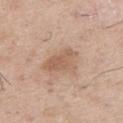* notes · catalogued during a skin exam; not biopsied
* illumination · white-light
* patient · male, roughly 50 years of age
* diameter · ~5 mm (longest diameter)
* automated metrics · a border-irregularity rating of about 3.5/10, internal color variation of about 3 on a 0–10 scale, and a peripheral color-asymmetry measure near 1; an automated nevus-likeness rating near 5 out of 100
* anatomic site · the right thigh
* imaging modality · ~15 mm crop, total-body skin-cancer survey